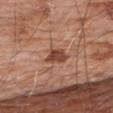workup = catalogued during a skin exam; not biopsied | location = the upper back | diameter = ≈3 mm | patient = male, aged approximately 80 | illumination = white-light illumination | imaging modality = ~15 mm tile from a whole-body skin photo.